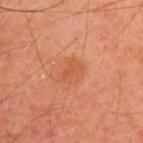Q: Was a biopsy performed?
A: imaged on a skin check; not biopsied
Q: What are the patient's age and sex?
A: male, aged 43 to 47
Q: Where on the body is the lesion?
A: the upper back
Q: How was this image acquired?
A: total-body-photography crop, ~15 mm field of view
Q: Automated lesion metrics?
A: a lesion area of about 6.5 mm² and a shape eccentricity near 0.55; roughly 5 lightness units darker than nearby skin and a normalized border contrast of about 5; a border-irregularity rating of about 3/10, a color-variation rating of about 2/10, and peripheral color asymmetry of about 0.5; a nevus-likeness score of about 0/100 and a lesion-detection confidence of about 100/100
Q: Illumination type?
A: cross-polarized illumination